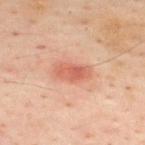Q: Was a biopsy performed?
A: catalogued during a skin exam; not biopsied
Q: What are the patient's age and sex?
A: male, approximately 50 years of age
Q: Where on the body is the lesion?
A: the upper back
Q: What kind of image is this?
A: 15 mm crop, total-body photography